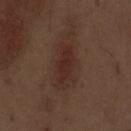<case>
<biopsy_status>not biopsied; imaged during a skin examination</biopsy_status>
<automated_metrics>
  <area_mm2_approx>9.0</area_mm2_approx>
  <eccentricity>0.95</eccentricity>
  <shape_asymmetry>0.25</shape_asymmetry>
  <cielab_L>28</cielab_L>
  <cielab_a>19</cielab_a>
  <cielab_b>22</cielab_b>
  <vs_skin_darker_L>7.0</vs_skin_darker_L>
  <vs_skin_contrast_norm>7.5</vs_skin_contrast_norm>
  <border_irregularity_0_10>4.0</border_irregularity_0_10>
  <color_variation_0_10>1.5</color_variation_0_10>
  <peripheral_color_asymmetry>0.5</peripheral_color_asymmetry>
</automated_metrics>
<lesion_size>
  <long_diameter_mm_approx>5.5</long_diameter_mm_approx>
</lesion_size>
<patient>
  <sex>male</sex>
  <age_approx>70</age_approx>
</patient>
<image>
  <source>total-body photography crop</source>
  <field_of_view_mm>15</field_of_view_mm>
</image>
<lighting>white-light</lighting>
<site>abdomen</site>
</case>